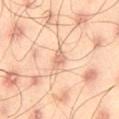Imaged with cross-polarized lighting. The recorded lesion diameter is about 2.5 mm. The total-body-photography lesion software estimated a mean CIELAB color near L≈70 a*≈22 b*≈32, roughly 10 lightness units darker than nearby skin, and a lesion-to-skin contrast of about 6 (normalized; higher = more distinct). The software also gave a border-irregularity rating of about 3/10. A roughly 15 mm field-of-view crop from a total-body skin photograph. From the right thigh. The patient is a male aged 43–47.